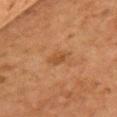biopsy status: no biopsy performed (imaged during a skin exam); tile lighting: cross-polarized illumination; subject: female, roughly 60 years of age; image: ~15 mm tile from a whole-body skin photo; size: ≈2.5 mm; site: the chest.